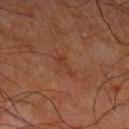  biopsy_status: not biopsied; imaged during a skin examination
  lesion_size:
    long_diameter_mm_approx: 3.0
  site: left upper arm
  patient:
    sex: male
    age_approx: 65
  lighting: cross-polarized
  image:
    source: total-body photography crop
    field_of_view_mm: 15